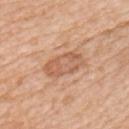Assessment: Recorded during total-body skin imaging; not selected for excision or biopsy. Acquisition and patient details: The lesion is located on the left upper arm. The patient is a female aged approximately 40. The lesion-visualizer software estimated a footprint of about 11 mm² and an eccentricity of roughly 0.85. The software also gave a border-irregularity index near 2.5/10, a within-lesion color-variation index near 3.5/10, and a peripheral color-asymmetry measure near 1. A roughly 15 mm field-of-view crop from a total-body skin photograph.